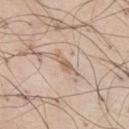{
  "biopsy_status": "not biopsied; imaged during a skin examination",
  "lighting": "white-light",
  "site": "right thigh",
  "image": {
    "source": "total-body photography crop",
    "field_of_view_mm": 15
  },
  "patient": {
    "sex": "male",
    "age_approx": 70
  }
}